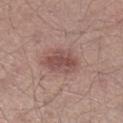| field | value |
|---|---|
| follow-up | catalogued during a skin exam; not biopsied |
| lighting | white-light |
| lesion size | about 5 mm |
| subject | male, approximately 40 years of age |
| location | the right lower leg |
| acquisition | ~15 mm crop, total-body skin-cancer survey |